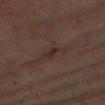notes = total-body-photography surveillance lesion; no biopsy
tile lighting = cross-polarized illumination
location = the left upper arm
patient = female, aged around 65
image = ~15 mm tile from a whole-body skin photo
TBP lesion metrics = a lesion color around L≈27 a*≈14 b*≈20 in CIELAB, a lesion–skin lightness drop of about 5, and a normalized border contrast of about 6.5
lesion size = about 3.5 mm